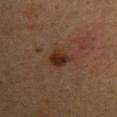notes — no biopsy performed (imaged during a skin exam)
image-analysis metrics — a border-irregularity rating of about 2/10
subject — female, roughly 40 years of age
body site — the left upper arm
tile lighting — cross-polarized
lesion diameter — ≈3 mm
acquisition — 15 mm crop, total-body photography